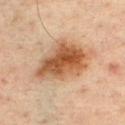Assessment: Imaged during a routine full-body skin examination; the lesion was not biopsied and no histopathology is available. Clinical summary: Imaged with cross-polarized lighting. The subject is a male aged 33–37. The lesion is on the chest. Longest diameter approximately 6.5 mm. A roughly 15 mm field-of-view crop from a total-body skin photograph.